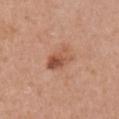This lesion was catalogued during total-body skin photography and was not selected for biopsy. The lesion is on the right upper arm. A 15 mm close-up tile from a total-body photography series done for melanoma screening. The tile uses white-light illumination. Measured at roughly 3.5 mm in maximum diameter. A female patient in their 50s.